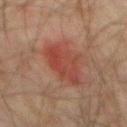Assessment: The lesion was photographed on a routine skin check and not biopsied; there is no pathology result. Clinical summary: A 15 mm close-up tile from a total-body photography series done for melanoma screening. A male patient approximately 75 years of age. Located on the abdomen.